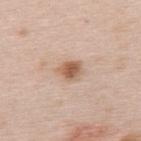- follow-up · total-body-photography surveillance lesion; no biopsy
- patient · female, aged 63–67
- image · total-body-photography crop, ~15 mm field of view
- site · the back